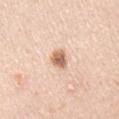Imaged during a routine full-body skin examination; the lesion was not biopsied and no histopathology is available. A region of skin cropped from a whole-body photographic capture, roughly 15 mm wide. From the right upper arm. The subject is a male aged 43 to 47.